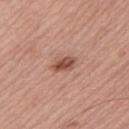Recorded during total-body skin imaging; not selected for excision or biopsy. A close-up tile cropped from a whole-body skin photograph, about 15 mm across. From the left upper arm. A male patient, aged around 70. The tile uses white-light illumination. Approximately 2.5 mm at its widest.